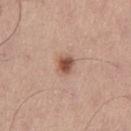lighting: white-light
automated lesion analysis: a lesion area of about 5 mm², an outline eccentricity of about 0.55 (0 = round, 1 = elongated), and a symmetry-axis asymmetry near 0.2; a mean CIELAB color near L≈54 a*≈21 b*≈28; a border-irregularity index near 2/10, internal color variation of about 4.5 on a 0–10 scale, and peripheral color asymmetry of about 1; an automated nevus-likeness rating near 95 out of 100 and a detector confidence of about 100 out of 100 that the crop contains a lesion
subject: male, aged 58–62
body site: the leg
acquisition: total-body-photography crop, ~15 mm field of view